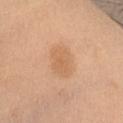<record>
<biopsy_status>not biopsied; imaged during a skin examination</biopsy_status>
<image>
  <source>total-body photography crop</source>
  <field_of_view_mm>15</field_of_view_mm>
</image>
<lighting>white-light</lighting>
<automated_metrics>
  <area_mm2_approx>10.0</area_mm2_approx>
  <eccentricity>0.7</eccentricity>
  <peripheral_color_asymmetry>0.5</peripheral_color_asymmetry>
</automated_metrics>
<patient>
  <sex>female</sex>
  <age_approx>45</age_approx>
</patient>
<site>chest</site>
<lesion_size>
  <long_diameter_mm_approx>4.0</long_diameter_mm_approx>
</lesion_size>
</record>